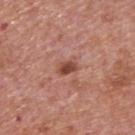{"biopsy_status": "not biopsied; imaged during a skin examination", "image": {"source": "total-body photography crop", "field_of_view_mm": 15}, "lighting": "white-light", "site": "upper back", "patient": {"sex": "male", "age_approx": 75}, "automated_metrics": {"cielab_L": 48, "cielab_a": 25, "cielab_b": 28, "vs_skin_darker_L": 11.0}, "lesion_size": {"long_diameter_mm_approx": 2.5}}